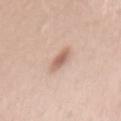Findings:
• workup · total-body-photography surveillance lesion; no biopsy
• diameter · about 3.5 mm
• site · the mid back
• automated metrics · a lesion color around L≈62 a*≈20 b*≈28 in CIELAB, roughly 12 lightness units darker than nearby skin, and a lesion-to-skin contrast of about 7.5 (normalized; higher = more distinct); a border-irregularity rating of about 3/10 and a color-variation rating of about 2.5/10; a detector confidence of about 100 out of 100 that the crop contains a lesion
• subject · female, in their mid- to late 30s
• acquisition · ~15 mm tile from a whole-body skin photo